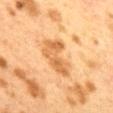This lesion was catalogued during total-body skin photography and was not selected for biopsy. From the mid back. A region of skin cropped from a whole-body photographic capture, roughly 15 mm wide. The patient is a female aged around 40.